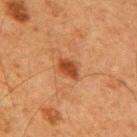Imaged during a routine full-body skin examination; the lesion was not biopsied and no histopathology is available. A close-up tile cropped from a whole-body skin photograph, about 15 mm across. Approximately 3 mm at its widest. A male patient, approximately 65 years of age. The lesion is located on the upper back. Imaged with cross-polarized lighting. The lesion-visualizer software estimated a footprint of about 4.5 mm², an outline eccentricity of about 0.75 (0 = round, 1 = elongated), and two-axis asymmetry of about 0.15. The analysis additionally found a normalized lesion–skin contrast near 9.